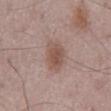{"biopsy_status": "not biopsied; imaged during a skin examination", "lighting": "white-light", "image": {"source": "total-body photography crop", "field_of_view_mm": 15}, "patient": {"sex": "male", "age_approx": 65}, "lesion_size": {"long_diameter_mm_approx": 4.5}, "site": "abdomen"}